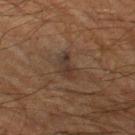{
  "biopsy_status": "not biopsied; imaged during a skin examination",
  "site": "left thigh",
  "patient": {
    "sex": "male",
    "age_approx": 65
  },
  "image": {
    "source": "total-body photography crop",
    "field_of_view_mm": 15
  }
}